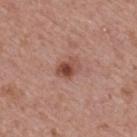Case summary:
• follow-up — imaged on a skin check; not biopsied
• location — the upper back
• subject — female, aged 63–67
• lesion size — ~3 mm (longest diameter)
• acquisition — ~15 mm crop, total-body skin-cancer survey
• image-analysis metrics — a mean CIELAB color near L≈48 a*≈24 b*≈27, a lesion–skin lightness drop of about 11, and a normalized lesion–skin contrast near 8.5; a border-irregularity rating of about 2.5/10, a within-lesion color-variation index near 7.5/10, and a peripheral color-asymmetry measure near 2.5
• illumination — white-light